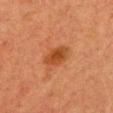The lesion is located on the chest. Cropped from a total-body skin-imaging series; the visible field is about 15 mm. A female patient, aged approximately 40. The recorded lesion diameter is about 3.5 mm.